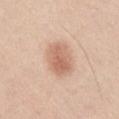<lesion>
  <patient>
    <sex>male</sex>
    <age_approx>60</age_approx>
  </patient>
  <site>abdomen</site>
  <image>
    <source>total-body photography crop</source>
    <field_of_view_mm>15</field_of_view_mm>
  </image>
</lesion>